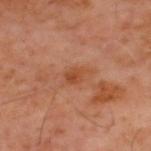location: the back
tile lighting: cross-polarized illumination
diameter: ≈3 mm
patient: male, aged 58 to 62
image source: 15 mm crop, total-body photography